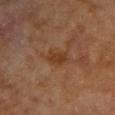A female subject aged approximately 80. The lesion is on the arm. A region of skin cropped from a whole-body photographic capture, roughly 15 mm wide. Longest diameter approximately 3 mm.